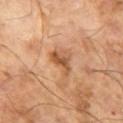Imaged during a routine full-body skin examination; the lesion was not biopsied and no histopathology is available. Measured at roughly 3.5 mm in maximum diameter. A 15 mm crop from a total-body photograph taken for skin-cancer surveillance. The tile uses cross-polarized illumination. The patient is a male aged approximately 65.